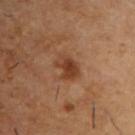Q: Is there a histopathology result?
A: total-body-photography surveillance lesion; no biopsy
Q: How large is the lesion?
A: about 3.5 mm
Q: What is the anatomic site?
A: the upper back
Q: Automated lesion metrics?
A: a mean CIELAB color near L≈39 a*≈22 b*≈32 and a normalized border contrast of about 8; a border-irregularity index near 2.5/10, internal color variation of about 4 on a 0–10 scale, and a peripheral color-asymmetry measure near 1.5
Q: Who is the patient?
A: male, about 55 years old
Q: Illumination type?
A: cross-polarized illumination
Q: How was this image acquired?
A: ~15 mm crop, total-body skin-cancer survey